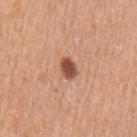Assessment: Imaged during a routine full-body skin examination; the lesion was not biopsied and no histopathology is available. Clinical summary: The lesion is on the left upper arm. The total-body-photography lesion software estimated a footprint of about 4 mm² and an eccentricity of roughly 0.7. The software also gave a mean CIELAB color near L≈51 a*≈25 b*≈31, a lesion–skin lightness drop of about 16, and a lesion-to-skin contrast of about 10.5 (normalized; higher = more distinct). It also reported a peripheral color-asymmetry measure near 0.5. The subject is a male aged 53–57. The recorded lesion diameter is about 2.5 mm. Captured under white-light illumination. A 15 mm close-up extracted from a 3D total-body photography capture.